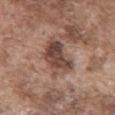follow-up: catalogued during a skin exam; not biopsied | tile lighting: white-light illumination | diameter: ~5.5 mm (longest diameter) | anatomic site: the back | image source: ~15 mm tile from a whole-body skin photo | subject: male, about 75 years old.